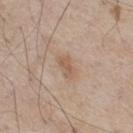{
  "lesion_size": {
    "long_diameter_mm_approx": 3.0
  },
  "site": "right thigh",
  "image": {
    "source": "total-body photography crop",
    "field_of_view_mm": 15
  },
  "patient": {
    "sex": "male",
    "age_approx": 60
  }
}